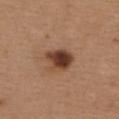follow-up = catalogued during a skin exam; not biopsied | subject = female, aged 38–42 | acquisition = total-body-photography crop, ~15 mm field of view | tile lighting = white-light illumination | image-analysis metrics = a footprint of about 8 mm², a shape eccentricity near 0.6, and a symmetry-axis asymmetry near 0.25; a border-irregularity rating of about 2/10, a color-variation rating of about 6.5/10, and a peripheral color-asymmetry measure near 2 | body site = the back | lesion diameter = ~3.5 mm (longest diameter).